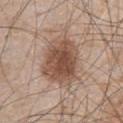{"biopsy_status": "not biopsied; imaged during a skin examination", "patient": {"sex": "male", "age_approx": 65}, "automated_metrics": {"area_mm2_approx": 23.0, "eccentricity": 0.6, "shape_asymmetry": 0.15, "border_irregularity_0_10": 2.0, "color_variation_0_10": 5.0, "peripheral_color_asymmetry": 1.5, "nevus_likeness_0_100": 95, "lesion_detection_confidence_0_100": 100}, "site": "abdomen", "image": {"source": "total-body photography crop", "field_of_view_mm": 15}, "lesion_size": {"long_diameter_mm_approx": 6.0}, "lighting": "white-light"}